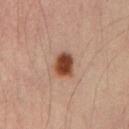From the right upper arm. Imaged with cross-polarized lighting. Automated tile analysis of the lesion measured a mean CIELAB color near L≈40 a*≈22 b*≈29, about 16 CIELAB-L* units darker than the surrounding skin, and a lesion-to-skin contrast of about 13 (normalized; higher = more distinct). The software also gave border irregularity of about 2 on a 0–10 scale, a color-variation rating of about 3.5/10, and a peripheral color-asymmetry measure near 1. A male subject aged around 35. A 15 mm close-up extracted from a 3D total-body photography capture.